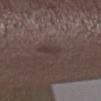Impression:
Imaged during a routine full-body skin examination; the lesion was not biopsied and no histopathology is available.
Image and clinical context:
The lesion is located on the right lower leg. The tile uses white-light illumination. The recorded lesion diameter is about 3.5 mm. This image is a 15 mm lesion crop taken from a total-body photograph. A female subject approximately 50 years of age.